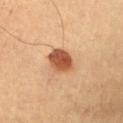Captured during whole-body skin photography for melanoma surveillance; the lesion was not biopsied. About 3.5 mm across. On the front of the torso. A 15 mm close-up extracted from a 3D total-body photography capture. A male patient, about 55 years old.